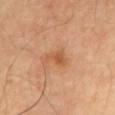This lesion was catalogued during total-body skin photography and was not selected for biopsy.
A region of skin cropped from a whole-body photographic capture, roughly 15 mm wide.
The lesion-visualizer software estimated a footprint of about 3.5 mm², an outline eccentricity of about 0.75 (0 = round, 1 = elongated), and two-axis asymmetry of about 0.55. The analysis additionally found a classifier nevus-likeness of about 0/100 and a lesion-detection confidence of about 100/100.
Captured under cross-polarized illumination.
A male patient aged around 65.
The lesion is on the chest.